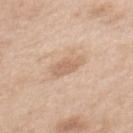workup = catalogued during a skin exam; not biopsied | subject = female, aged around 40 | image = ~15 mm crop, total-body skin-cancer survey | location = the mid back | lighting = white-light illumination.